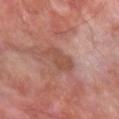The lesion was tiled from a total-body skin photograph and was not biopsied. Cropped from a total-body skin-imaging series; the visible field is about 15 mm. The subject is a male aged 63–67. Located on the left forearm.